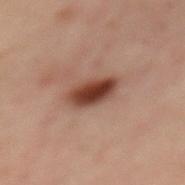| key | value |
|---|---|
| workup | catalogued during a skin exam; not biopsied |
| patient | female, aged approximately 55 |
| diameter | ~4 mm (longest diameter) |
| site | the mid back |
| acquisition | total-body-photography crop, ~15 mm field of view |
| lighting | cross-polarized |